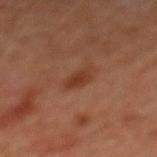Assessment: This lesion was catalogued during total-body skin photography and was not selected for biopsy.